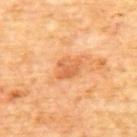Part of a total-body skin-imaging series; this lesion was reviewed on a skin check and was not flagged for biopsy. Captured under cross-polarized illumination. The lesion-visualizer software estimated an outline eccentricity of about 0.7 (0 = round, 1 = elongated) and two-axis asymmetry of about 0.2. The analysis additionally found an average lesion color of about L≈62 a*≈28 b*≈43 (CIELAB) and roughly 9 lightness units darker than nearby skin. Cropped from a whole-body photographic skin survey; the tile spans about 15 mm. The lesion is located on the mid back. A male subject roughly 60 years of age.